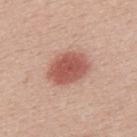No biopsy was performed on this lesion — it was imaged during a full skin examination and was not determined to be concerning.
The lesion's longest dimension is about 5 mm.
A male patient aged approximately 40.
This image is a 15 mm lesion crop taken from a total-body photograph.
This is a white-light tile.
Located on the back.